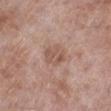Part of a total-body skin-imaging series; this lesion was reviewed on a skin check and was not flagged for biopsy.
A close-up tile cropped from a whole-body skin photograph, about 15 mm across.
Located on the right lower leg.
The total-body-photography lesion software estimated an area of roughly 7 mm² and a shape eccentricity near 0.55. The analysis additionally found a border-irregularity rating of about 1.5/10 and internal color variation of about 3.5 on a 0–10 scale.
The tile uses white-light illumination.
A male patient, roughly 75 years of age.
Measured at roughly 3 mm in maximum diameter.